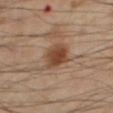{"lesion_size": {"long_diameter_mm_approx": 4.0}, "patient": {"sex": "male", "age_approx": 55}, "image": {"source": "total-body photography crop", "field_of_view_mm": 15}, "automated_metrics": {"area_mm2_approx": 10.0, "eccentricity": 0.7, "nevus_likeness_0_100": 95}, "site": "right thigh", "lighting": "cross-polarized"}